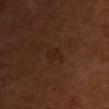Background: Approximately 2.5 mm at its widest. The subject is a female in their mid-50s. Cropped from a whole-body photographic skin survey; the tile spans about 15 mm. Imaged with cross-polarized lighting. The lesion is on the chest.